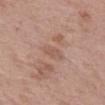biopsy status — imaged on a skin check; not biopsied
anatomic site — the abdomen
subject — female, approximately 40 years of age
acquisition — 15 mm crop, total-body photography
size — about 2.5 mm
lighting — white-light